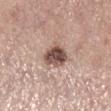Q: Is there a histopathology result?
A: catalogued during a skin exam; not biopsied
Q: What lighting was used for the tile?
A: white-light
Q: Lesion size?
A: ≈3.5 mm
Q: What are the patient's age and sex?
A: female, about 65 years old
Q: What is the imaging modality?
A: 15 mm crop, total-body photography
Q: Where on the body is the lesion?
A: the right lower leg